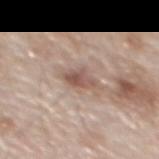Notes:
– notes · imaged on a skin check; not biopsied
– automated lesion analysis · a footprint of about 5 mm²; a mean CIELAB color near L≈54 a*≈18 b*≈24, a lesion–skin lightness drop of about 11, and a lesion-to-skin contrast of about 7.5 (normalized; higher = more distinct)
– illumination · white-light illumination
– patient · male, about 80 years old
– image source · 15 mm crop, total-body photography
– site · the mid back